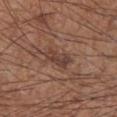Imaged during a routine full-body skin examination; the lesion was not biopsied and no histopathology is available. From the right forearm. Measured at roughly 3.5 mm in maximum diameter. A male subject aged approximately 55. A roughly 15 mm field-of-view crop from a total-body skin photograph. Automated image analysis of the tile measured an area of roughly 6 mm², a shape eccentricity near 0.85, and a shape-asymmetry score of about 0.25 (0 = symmetric). And it measured a mean CIELAB color near L≈41 a*≈19 b*≈25, a lesion–skin lightness drop of about 8, and a lesion-to-skin contrast of about 7 (normalized; higher = more distinct). It also reported a nevus-likeness score of about 0/100 and lesion-presence confidence of about 85/100. Imaged with white-light lighting.